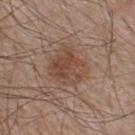A male subject in their mid- to late 50s. A region of skin cropped from a whole-body photographic capture, roughly 15 mm wide. Approximately 4 mm at its widest. On the upper back. The tile uses white-light illumination.